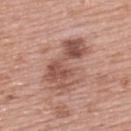Imaged with white-light lighting.
Automated image analysis of the tile measured a lesion area of about 19 mm², an eccentricity of roughly 0.9, and a symmetry-axis asymmetry near 0.45. The analysis additionally found a lesion color around L≈53 a*≈23 b*≈26 in CIELAB, roughly 12 lightness units darker than nearby skin, and a normalized lesion–skin contrast near 8. It also reported internal color variation of about 6 on a 0–10 scale and peripheral color asymmetry of about 2. The software also gave an automated nevus-likeness rating near 30 out of 100 and a lesion-detection confidence of about 100/100.
On the upper back.
The patient is a female about 60 years old.
The recorded lesion diameter is about 7 mm.
Cropped from a whole-body photographic skin survey; the tile spans about 15 mm.